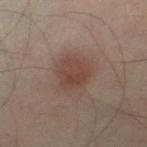This lesion was catalogued during total-body skin photography and was not selected for biopsy.
A close-up tile cropped from a whole-body skin photograph, about 15 mm across.
The lesion-visualizer software estimated a mean CIELAB color near L≈40 a*≈18 b*≈23.
Measured at roughly 3.5 mm in maximum diameter.
From the leg.
A male patient roughly 35 years of age.
Captured under cross-polarized illumination.